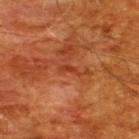Q: What is the imaging modality?
A: 15 mm crop, total-body photography
Q: How large is the lesion?
A: ~3 mm (longest diameter)
Q: Where on the body is the lesion?
A: the upper back
Q: Who is the patient?
A: male, approximately 60 years of age